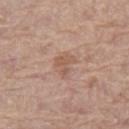Clinical impression: Imaged during a routine full-body skin examination; the lesion was not biopsied and no histopathology is available. Acquisition and patient details: This is a white-light tile. The lesion is located on the left thigh. Cropped from a total-body skin-imaging series; the visible field is about 15 mm. The patient is a female in their mid- to late 60s. The lesion's longest dimension is about 2.5 mm.